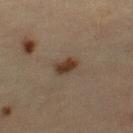Q: Was a biopsy performed?
A: total-body-photography surveillance lesion; no biopsy
Q: What is the lesion's diameter?
A: about 2.5 mm
Q: Patient demographics?
A: female, aged 63–67
Q: Illumination type?
A: cross-polarized
Q: How was this image acquired?
A: ~15 mm tile from a whole-body skin photo
Q: What is the anatomic site?
A: the right thigh
Q: What did automated image analysis measure?
A: a mean CIELAB color near L≈31 a*≈13 b*≈24 and a lesion–skin lightness drop of about 10; a border-irregularity index near 2/10 and radial color variation of about 0.5; an automated nevus-likeness rating near 95 out of 100 and a lesion-detection confidence of about 100/100